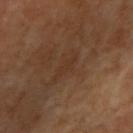Captured during whole-body skin photography for melanoma surveillance; the lesion was not biopsied.
The total-body-photography lesion software estimated a mean CIELAB color near L≈34 a*≈18 b*≈29, a lesion–skin lightness drop of about 4, and a normalized lesion–skin contrast near 4.5.
Cropped from a total-body skin-imaging series; the visible field is about 15 mm.
The tile uses cross-polarized illumination.
The lesion's longest dimension is about 2.5 mm.
From the right upper arm.